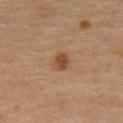Recorded during total-body skin imaging; not selected for excision or biopsy. The subject is a male aged approximately 75. This is a cross-polarized tile. This image is a 15 mm lesion crop taken from a total-body photograph. About 2.5 mm across. The lesion is located on the abdomen.